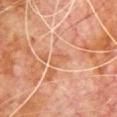Q: Is there a histopathology result?
A: no biopsy performed (imaged during a skin exam)
Q: What are the patient's age and sex?
A: male, aged around 80
Q: What lighting was used for the tile?
A: cross-polarized
Q: Where on the body is the lesion?
A: the chest
Q: What kind of image is this?
A: ~15 mm crop, total-body skin-cancer survey
Q: How large is the lesion?
A: about 3 mm
Q: What did automated image analysis measure?
A: an eccentricity of roughly 0.7 and two-axis asymmetry of about 0.3; a lesion color around L≈47 a*≈20 b*≈30 in CIELAB, roughly 5 lightness units darker than nearby skin, and a normalized lesion–skin contrast near 5; border irregularity of about 3 on a 0–10 scale and a peripheral color-asymmetry measure near 1